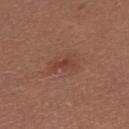The lesion is on the back. Imaged with white-light lighting. The recorded lesion diameter is about 3 mm. A female subject, aged 23–27. Cropped from a total-body skin-imaging series; the visible field is about 15 mm.